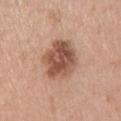The lesion was photographed on a routine skin check and not biopsied; there is no pathology result.
The patient is a female aged around 45.
The lesion's longest dimension is about 5.5 mm.
A close-up tile cropped from a whole-body skin photograph, about 15 mm across.
Located on the right upper arm.